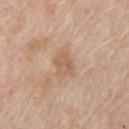biopsy_status: not biopsied; imaged during a skin examination
lesion_size:
  long_diameter_mm_approx: 3.0
site: front of the torso
patient:
  sex: male
  age_approx: 65
automated_metrics:
  area_mm2_approx: 6.5
  eccentricity: 0.7
  shape_asymmetry: 0.25
  cielab_L: 60
  cielab_a: 17
  cielab_b: 32
  vs_skin_darker_L: 7.0
  vs_skin_contrast_norm: 5.5
  nevus_likeness_0_100: 0
  lesion_detection_confidence_0_100: 100
lighting: white-light
image:
  source: total-body photography crop
  field_of_view_mm: 15